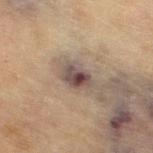Q: Is there a histopathology result?
A: total-body-photography surveillance lesion; no biopsy
Q: What lighting was used for the tile?
A: cross-polarized
Q: What did automated image analysis measure?
A: a lesion area of about 7 mm² and a shape-asymmetry score of about 0.25 (0 = symmetric); about 11 CIELAB-L* units darker than the surrounding skin and a normalized border contrast of about 10.5; a border-irregularity index near 2.5/10, a within-lesion color-variation index near 8/10, and peripheral color asymmetry of about 2.5; an automated nevus-likeness rating near 50 out of 100 and a detector confidence of about 100 out of 100 that the crop contains a lesion
Q: Who is the patient?
A: female, roughly 80 years of age
Q: What is the lesion's diameter?
A: ~3.5 mm (longest diameter)
Q: What is the anatomic site?
A: the right thigh
Q: What kind of image is this?
A: 15 mm crop, total-body photography